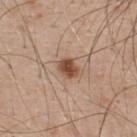| field | value |
|---|---|
| notes | catalogued during a skin exam; not biopsied |
| lesion diameter | about 3 mm |
| image-analysis metrics | a shape eccentricity near 0.65 and a symmetry-axis asymmetry near 0.2; an average lesion color of about L≈50 a*≈21 b*≈30 (CIELAB) and a lesion-to-skin contrast of about 10 (normalized; higher = more distinct); internal color variation of about 3.5 on a 0–10 scale |
| location | the upper back |
| subject | male, aged 48 to 52 |
| acquisition | total-body-photography crop, ~15 mm field of view |
| lighting | white-light illumination |